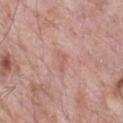<lesion>
<biopsy_status>not biopsied; imaged during a skin examination</biopsy_status>
<site>chest</site>
<patient>
  <sex>male</sex>
  <age_approx>70</age_approx>
</patient>
<image>
  <source>total-body photography crop</source>
  <field_of_view_mm>15</field_of_view_mm>
</image>
<lesion_size>
  <long_diameter_mm_approx>2.5</long_diameter_mm_approx>
</lesion_size>
<automated_metrics>
  <area_mm2_approx>2.5</area_mm2_approx>
  <vs_skin_darker_L>6.0</vs_skin_darker_L>
  <border_irregularity_0_10>4.0</border_irregularity_0_10>
  <color_variation_0_10>0.0</color_variation_0_10>
  <nevus_likeness_0_100>0</nevus_likeness_0_100>
  <lesion_detection_confidence_0_100>100</lesion_detection_confidence_0_100>
</automated_metrics>
</lesion>